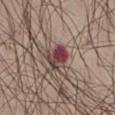Part of a total-body skin-imaging series; this lesion was reviewed on a skin check and was not flagged for biopsy. A male subject, approximately 55 years of age. A 15 mm crop from a total-body photograph taken for skin-cancer surveillance. On the right thigh.